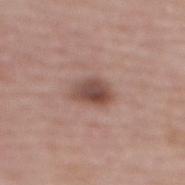Impression: Recorded during total-body skin imaging; not selected for excision or biopsy. Image and clinical context: A 15 mm crop from a total-body photograph taken for skin-cancer surveillance. A female subject, in their mid-60s. The lesion is located on the back.